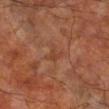{
  "biopsy_status": "not biopsied; imaged during a skin examination",
  "site": "right lower leg",
  "lesion_size": {
    "long_diameter_mm_approx": 2.5
  },
  "patient": {
    "sex": "male",
    "age_approx": 60
  },
  "lighting": "cross-polarized",
  "image": {
    "source": "total-body photography crop",
    "field_of_view_mm": 15
  },
  "automated_metrics": {
    "vs_skin_darker_L": 5.0
  }
}